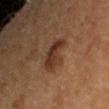notes = imaged on a skin check; not biopsied
patient = female, aged 53–57
lesion diameter = ≈3.5 mm
lighting = cross-polarized
anatomic site = the right upper arm
acquisition = ~15 mm tile from a whole-body skin photo